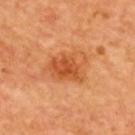Recorded during total-body skin imaging; not selected for excision or biopsy.